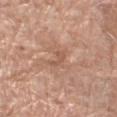Recorded during total-body skin imaging; not selected for excision or biopsy.
The lesion is located on the left thigh.
Imaged with white-light lighting.
Longest diameter approximately 2.5 mm.
This image is a 15 mm lesion crop taken from a total-body photograph.
A female patient aged 73 to 77.
An algorithmic analysis of the crop reported a border-irregularity index near 5.5/10, internal color variation of about 0 on a 0–10 scale, and a peripheral color-asymmetry measure near 0. The software also gave a classifier nevus-likeness of about 0/100 and a detector confidence of about 75 out of 100 that the crop contains a lesion.